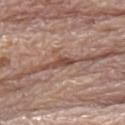The lesion was tiled from a total-body skin photograph and was not biopsied.
Cropped from a total-body skin-imaging series; the visible field is about 15 mm.
From the right lower leg.
The total-body-photography lesion software estimated a border-irregularity index near 4/10, internal color variation of about 0 on a 0–10 scale, and a peripheral color-asymmetry measure near 0. And it measured a classifier nevus-likeness of about 0/100 and lesion-presence confidence of about 75/100.
Approximately 3 mm at its widest.
This is a white-light tile.
A male patient approximately 70 years of age.